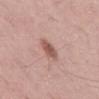Recorded during total-body skin imaging; not selected for excision or biopsy. A male patient roughly 55 years of age. The lesion is on the front of the torso. Measured at roughly 3 mm in maximum diameter. Cropped from a total-body skin-imaging series; the visible field is about 15 mm.